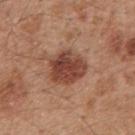biopsy status: no biopsy performed (imaged during a skin exam)
site: the mid back
lighting: white-light
image-analysis metrics: an area of roughly 13 mm², an outline eccentricity of about 0.45 (0 = round, 1 = elongated), and a symmetry-axis asymmetry near 0.2; a lesion color around L≈44 a*≈24 b*≈28 in CIELAB and about 13 CIELAB-L* units darker than the surrounding skin; a border-irregularity index near 1.5/10, a color-variation rating of about 4.5/10, and peripheral color asymmetry of about 1.5
lesion diameter: ≈4.5 mm
image source: total-body-photography crop, ~15 mm field of view
subject: male, roughly 45 years of age